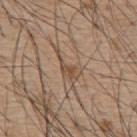follow-up = no biopsy performed (imaged during a skin exam); subject = male, aged 53 to 57; acquisition = 15 mm crop, total-body photography; site = the upper back.